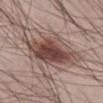Recorded during total-body skin imaging; not selected for excision or biopsy.